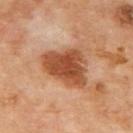{
  "biopsy_status": "not biopsied; imaged during a skin examination",
  "lighting": "cross-polarized",
  "image": {
    "source": "total-body photography crop",
    "field_of_view_mm": 15
  },
  "patient": {
    "sex": "male",
    "age_approx": 70
  },
  "lesion_size": {
    "long_diameter_mm_approx": 6.0
  },
  "automated_metrics": {
    "cielab_L": 50,
    "cielab_a": 25,
    "cielab_b": 36,
    "vs_skin_darker_L": 14.0,
    "border_irregularity_0_10": 3.5,
    "peripheral_color_asymmetry": 1.0
  },
  "site": "mid back"
}